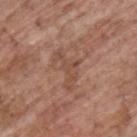Recorded during total-body skin imaging; not selected for excision or biopsy. A 15 mm close-up tile from a total-body photography series done for melanoma screening. A male subject, roughly 70 years of age. From the upper back. Imaged with white-light lighting.